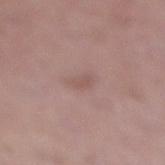Q: Was this lesion biopsied?
A: catalogued during a skin exam; not biopsied
Q: Lesion size?
A: about 2.5 mm
Q: What is the anatomic site?
A: the right lower leg
Q: What did automated image analysis measure?
A: a footprint of about 2.5 mm², an outline eccentricity of about 0.85 (0 = round, 1 = elongated), and a symmetry-axis asymmetry near 0.35; roughly 6 lightness units darker than nearby skin and a normalized border contrast of about 4.5; a peripheral color-asymmetry measure near 0
Q: Illumination type?
A: white-light illumination
Q: Patient demographics?
A: female, aged around 40
Q: What kind of image is this?
A: total-body-photography crop, ~15 mm field of view